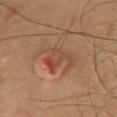Assessment: The lesion was photographed on a routine skin check and not biopsied; there is no pathology result. Clinical summary: The lesion is on the front of the torso. Captured under cross-polarized illumination. Approximately 5 mm at its widest. Automated image analysis of the tile measured a lesion area of about 9.5 mm², an outline eccentricity of about 0.75 (0 = round, 1 = elongated), and a symmetry-axis asymmetry near 0.4. The software also gave a lesion–skin lightness drop of about 7 and a normalized border contrast of about 5.5. The software also gave a border-irregularity index near 6/10. It also reported an automated nevus-likeness rating near 0 out of 100. The subject is a male approximately 60 years of age. A 15 mm close-up tile from a total-body photography series done for melanoma screening.